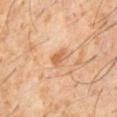* workup: catalogued during a skin exam; not biopsied
* lesion size: ≈2.5 mm
* acquisition: total-body-photography crop, ~15 mm field of view
* patient: male, roughly 60 years of age
* illumination: cross-polarized illumination
* body site: the front of the torso